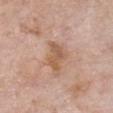Assessment:
No biopsy was performed on this lesion — it was imaged during a full skin examination and was not determined to be concerning.
Clinical summary:
Measured at roughly 4 mm in maximum diameter. On the front of the torso. The tile uses white-light illumination. A lesion tile, about 15 mm wide, cut from a 3D total-body photograph. A female patient, in their mid-70s. The lesion-visualizer software estimated a classifier nevus-likeness of about 0/100 and a lesion-detection confidence of about 100/100.